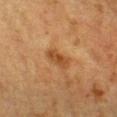Q: Was this lesion biopsied?
A: catalogued during a skin exam; not biopsied
Q: What are the patient's age and sex?
A: female, aged around 55
Q: What is the imaging modality?
A: ~15 mm crop, total-body skin-cancer survey
Q: What did automated image analysis measure?
A: an area of roughly 4.5 mm², a shape eccentricity near 0.85, and a shape-asymmetry score of about 0.25 (0 = symmetric); a lesion color around L≈39 a*≈21 b*≈34 in CIELAB, about 8 CIELAB-L* units darker than the surrounding skin, and a normalized lesion–skin contrast near 7.5; a color-variation rating of about 2.5/10
Q: How large is the lesion?
A: about 3 mm
Q: How was the tile lit?
A: cross-polarized
Q: Lesion location?
A: the head or neck